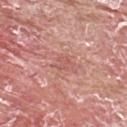Case summary:
– follow-up · catalogued during a skin exam; not biopsied
– lesion diameter · about 2.5 mm
– illumination · white-light
– site · the back
– image · total-body-photography crop, ~15 mm field of view
– patient · male, about 65 years old